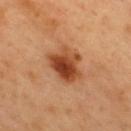Recorded during total-body skin imaging; not selected for excision or biopsy. The lesion is on the upper back. The tile uses cross-polarized illumination. A close-up tile cropped from a whole-body skin photograph, about 15 mm across. A male patient aged approximately 50.